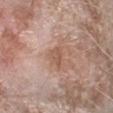Notes:
- notes — no biopsy performed (imaged during a skin exam)
- subject — female, about 75 years old
- body site — the right forearm
- imaging modality — ~15 mm tile from a whole-body skin photo
- tile lighting — white-light illumination
- size — ~2.5 mm (longest diameter)